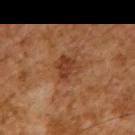Assessment: Part of a total-body skin-imaging series; this lesion was reviewed on a skin check and was not flagged for biopsy. Background: The lesion's longest dimension is about 3 mm. Imaged with cross-polarized lighting. This image is a 15 mm lesion crop taken from a total-body photograph. The patient is a male approximately 65 years of age.